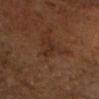notes: imaged on a skin check; not biopsied
size: ~2.5 mm (longest diameter)
illumination: cross-polarized
patient: male, in their mid-60s
image source: total-body-photography crop, ~15 mm field of view
body site: the head or neck